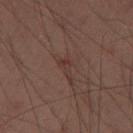The subject is a male aged 38–42.
About 3 mm across.
Imaged with cross-polarized lighting.
From the left thigh.
A region of skin cropped from a whole-body photographic capture, roughly 15 mm wide.
The total-body-photography lesion software estimated a mean CIELAB color near L≈26 a*≈14 b*≈17, about 4 CIELAB-L* units darker than the surrounding skin, and a lesion-to-skin contrast of about 5 (normalized; higher = more distinct). And it measured a border-irregularity index near 6.5/10, a within-lesion color-variation index near 0.5/10, and a peripheral color-asymmetry measure near 0.